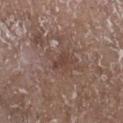Captured under white-light illumination. The subject is a male aged approximately 65. A 15 mm close-up extracted from a 3D total-body photography capture. From the right lower leg. Measured at roughly 2.5 mm in maximum diameter.